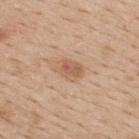Clinical impression:
The lesion was photographed on a routine skin check and not biopsied; there is no pathology result.
Clinical summary:
Imaged with white-light lighting. Cropped from a total-body skin-imaging series; the visible field is about 15 mm. A male subject aged 58–62. From the back.